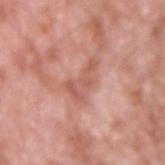Located on the right upper arm. Approximately 4 mm at its widest. A male subject, aged 58 to 62. Automated tile analysis of the lesion measured an area of roughly 7 mm² and an outline eccentricity of about 0.85 (0 = round, 1 = elongated). It also reported border irregularity of about 6.5 on a 0–10 scale, internal color variation of about 2.5 on a 0–10 scale, and radial color variation of about 0.5. Captured under white-light illumination. A 15 mm crop from a total-body photograph taken for skin-cancer surveillance.